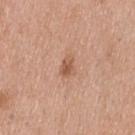Findings:
• site — the back
• subject — male, approximately 40 years of age
• size — ≈2.5 mm
• illumination — white-light illumination
• image — ~15 mm tile from a whole-body skin photo